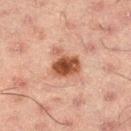Recorded during total-body skin imaging; not selected for excision or biopsy. From the left thigh. Approximately 3.5 mm at its widest. Imaged with cross-polarized lighting. A 15 mm close-up tile from a total-body photography series done for melanoma screening. A male patient, aged 48 to 52.